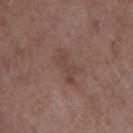This lesion was catalogued during total-body skin photography and was not selected for biopsy.
A region of skin cropped from a whole-body photographic capture, roughly 15 mm wide.
Captured under white-light illumination.
From the right upper arm.
A male subject, in their 50s.